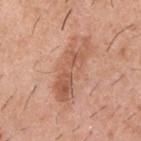No biopsy was performed on this lesion — it was imaged during a full skin examination and was not determined to be concerning. A 15 mm close-up extracted from a 3D total-body photography capture. The lesion is located on the upper back. The subject is a male approximately 40 years of age.